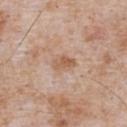Impression:
This lesion was catalogued during total-body skin photography and was not selected for biopsy.
Acquisition and patient details:
This is a white-light tile. The patient is a male in their mid- to late 60s. On the front of the torso. A 15 mm close-up extracted from a 3D total-body photography capture.